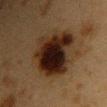Assessment:
No biopsy was performed on this lesion — it was imaged during a full skin examination and was not determined to be concerning.
Context:
The recorded lesion diameter is about 6 mm. A region of skin cropped from a whole-body photographic capture, roughly 15 mm wide. The subject is a female roughly 40 years of age. On the chest. Imaged with cross-polarized lighting.